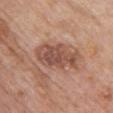The lesion was tiled from a total-body skin photograph and was not biopsied.
A region of skin cropped from a whole-body photographic capture, roughly 15 mm wide.
A female subject, approximately 60 years of age.
The lesion is on the chest.